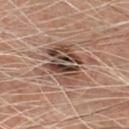Part of a total-body skin-imaging series; this lesion was reviewed on a skin check and was not flagged for biopsy.
The lesion is on the chest.
Captured under cross-polarized illumination.
A close-up tile cropped from a whole-body skin photograph, about 15 mm across.
The lesion-visualizer software estimated a nevus-likeness score of about 45/100.
The lesion's longest dimension is about 5.5 mm.
A male patient aged 63 to 67.